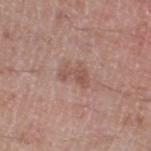The lesion was tiled from a total-body skin photograph and was not biopsied. Automated tile analysis of the lesion measured a shape eccentricity near 0.8 and a shape-asymmetry score of about 0.45 (0 = symmetric). And it measured a mean CIELAB color near L≈53 a*≈20 b*≈25 and a lesion-to-skin contrast of about 6 (normalized; higher = more distinct). The analysis additionally found a border-irregularity rating of about 5/10, a within-lesion color-variation index near 2.5/10, and radial color variation of about 0.5. The analysis additionally found a nevus-likeness score of about 0/100 and a detector confidence of about 100 out of 100 that the crop contains a lesion. Captured under white-light illumination. A male patient aged 48–52. A 15 mm close-up extracted from a 3D total-body photography capture. The lesion's longest dimension is about 3.5 mm. From the left lower leg.